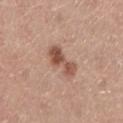Clinical impression:
Imaged during a routine full-body skin examination; the lesion was not biopsied and no histopathology is available.
Clinical summary:
Longest diameter approximately 4 mm. On the left lower leg. A 15 mm close-up tile from a total-body photography series done for melanoma screening. A female patient approximately 45 years of age. The tile uses white-light illumination.